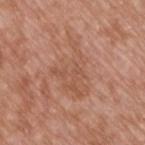workup = total-body-photography surveillance lesion; no biopsy | subject = male, approximately 50 years of age | anatomic site = the upper back | imaging modality = total-body-photography crop, ~15 mm field of view | lighting = white-light illumination.